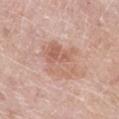Captured during whole-body skin photography for melanoma surveillance; the lesion was not biopsied. Approximately 5 mm at its widest. The patient is a female in their 70s. The lesion-visualizer software estimated a lesion area of about 14 mm² and an outline eccentricity of about 0.45 (0 = round, 1 = elongated). It also reported a lesion–skin lightness drop of about 8 and a normalized border contrast of about 6. It also reported a border-irregularity index near 5/10, a color-variation rating of about 6/10, and a peripheral color-asymmetry measure near 2. On the left lower leg. The tile uses white-light illumination. A 15 mm close-up extracted from a 3D total-body photography capture.